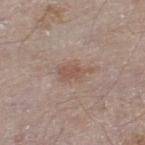A male patient roughly 65 years of age. The lesion is on the left thigh. Cropped from a whole-body photographic skin survey; the tile spans about 15 mm.